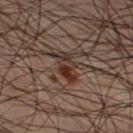The tile uses cross-polarized illumination.
This image is a 15 mm lesion crop taken from a total-body photograph.
Automated image analysis of the tile measured border irregularity of about 4.5 on a 0–10 scale, internal color variation of about 6.5 on a 0–10 scale, and peripheral color asymmetry of about 2. The analysis additionally found a detector confidence of about 100 out of 100 that the crop contains a lesion.
The lesion is on the right lower leg.
About 3.5 mm across.
A male patient aged around 50.